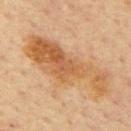follow-up: total-body-photography surveillance lesion; no biopsy
patient: male, aged approximately 65
acquisition: 15 mm crop, total-body photography
location: the mid back